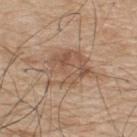Notes:
– biopsy status · imaged on a skin check; not biopsied
– lighting · white-light
– lesion size · ~5.5 mm (longest diameter)
– patient · male, approximately 75 years of age
– imaging modality · 15 mm crop, total-body photography
– automated metrics · a nevus-likeness score of about 50/100 and a detector confidence of about 100 out of 100 that the crop contains a lesion
– site · the upper back